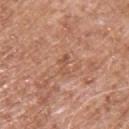Captured under white-light illumination.
The lesion is on the upper back.
Cropped from a total-body skin-imaging series; the visible field is about 15 mm.
The subject is a male in their mid- to late 50s.
The lesion's longest dimension is about 2.5 mm.